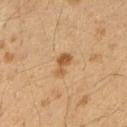biopsy status: imaged on a skin check; not biopsied | lesion diameter: ≈3 mm | body site: the right upper arm | tile lighting: cross-polarized illumination | patient: female, aged 38–42 | acquisition: ~15 mm crop, total-body skin-cancer survey.